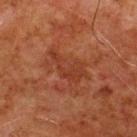Q: How was this image acquired?
A: ~15 mm crop, total-body skin-cancer survey
Q: What is the anatomic site?
A: the upper back
Q: What are the patient's age and sex?
A: male, in their 80s